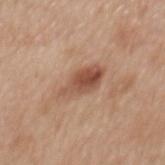notes = imaged on a skin check; not biopsied
anatomic site = the mid back
patient = female, aged 38–42
image source = ~15 mm tile from a whole-body skin photo
illumination = white-light